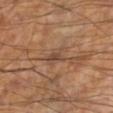Part of a total-body skin-imaging series; this lesion was reviewed on a skin check and was not flagged for biopsy. The lesion is on the left lower leg. The tile uses cross-polarized illumination. A 15 mm close-up extracted from a 3D total-body photography capture. The patient is a male aged 58–62. The lesion's longest dimension is about 3 mm. Automated image analysis of the tile measured an eccentricity of roughly 0.7 and two-axis asymmetry of about 0.35. And it measured a mean CIELAB color near L≈45 a*≈17 b*≈27, roughly 7 lightness units darker than nearby skin, and a lesion-to-skin contrast of about 6 (normalized; higher = more distinct). The analysis additionally found a within-lesion color-variation index near 3.5/10 and radial color variation of about 1.5.